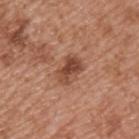Q: Was a biopsy performed?
A: no biopsy performed (imaged during a skin exam)
Q: Who is the patient?
A: male, in their mid- to late 50s
Q: Illumination type?
A: white-light
Q: Lesion location?
A: the upper back
Q: What is the lesion's diameter?
A: ≈4 mm
Q: What is the imaging modality?
A: 15 mm crop, total-body photography
Q: Automated lesion metrics?
A: a border-irregularity rating of about 2/10 and internal color variation of about 6 on a 0–10 scale; an automated nevus-likeness rating near 70 out of 100 and a lesion-detection confidence of about 100/100